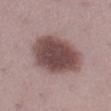Captured during whole-body skin photography for melanoma surveillance; the lesion was not biopsied. The lesion-visualizer software estimated a lesion area of about 27 mm², a shape eccentricity near 0.8, and two-axis asymmetry of about 0.1. It also reported a lesion color around L≈46 a*≈19 b*≈18 in CIELAB, roughly 16 lightness units darker than nearby skin, and a normalized border contrast of about 11.5. Imaged with white-light lighting. The patient is a female aged around 25. The recorded lesion diameter is about 7.5 mm. The lesion is located on the right lower leg. A 15 mm close-up tile from a total-body photography series done for melanoma screening.